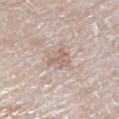The lesion was photographed on a routine skin check and not biopsied; there is no pathology result. Measured at roughly 3 mm in maximum diameter. A male subject, approximately 80 years of age. The total-body-photography lesion software estimated a lesion area of about 4.5 mm² and a symmetry-axis asymmetry near 0.45. And it measured a border-irregularity rating of about 4.5/10. The software also gave a classifier nevus-likeness of about 0/100 and a lesion-detection confidence of about 55/100. Imaged with white-light lighting. The lesion is located on the left lower leg. This image is a 15 mm lesion crop taken from a total-body photograph.